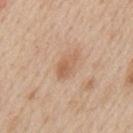workup: no biopsy performed (imaged during a skin exam) | image-analysis metrics: a lesion color around L≈60 a*≈19 b*≈33 in CIELAB and a lesion–skin lightness drop of about 8; a classifier nevus-likeness of about 0/100 | acquisition: 15 mm crop, total-body photography | tile lighting: white-light | patient: male, about 60 years old | location: the mid back | size: ~3.5 mm (longest diameter).